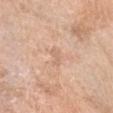illumination = white-light illumination; anatomic site = the head or neck; patient = female, aged around 55; diameter = ≈2.5 mm; imaging modality = total-body-photography crop, ~15 mm field of view.